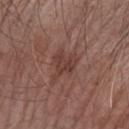follow-up — no biopsy performed (imaged during a skin exam) | size — about 3.5 mm | image-analysis metrics — an average lesion color of about L≈39 a*≈21 b*≈22 (CIELAB) and a lesion-to-skin contrast of about 6.5 (normalized; higher = more distinct); border irregularity of about 5 on a 0–10 scale and radial color variation of about 0.5; a nevus-likeness score of about 15/100 | body site — the left forearm | image — ~15 mm crop, total-body skin-cancer survey | patient — male, aged 73–77.